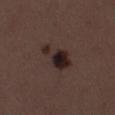{
  "biopsy_status": "not biopsied; imaged during a skin examination",
  "automated_metrics": {
    "lesion_detection_confidence_0_100": 100
  },
  "image": {
    "source": "total-body photography crop",
    "field_of_view_mm": 15
  },
  "patient": {
    "sex": "female",
    "age_approx": 50
  },
  "lesion_size": {
    "long_diameter_mm_approx": 4.5
  },
  "site": "right thigh",
  "lighting": "white-light"
}